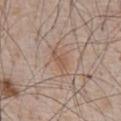Impression: Imaged during a routine full-body skin examination; the lesion was not biopsied and no histopathology is available. Image and clinical context: A male subject aged 63–67. Automated image analysis of the tile measured a footprint of about 4 mm² and a shape-asymmetry score of about 0.5 (0 = symmetric). It also reported a border-irregularity index near 5.5/10, a within-lesion color-variation index near 1.5/10, and radial color variation of about 0.5. It also reported an automated nevus-likeness rating near 15 out of 100 and a detector confidence of about 100 out of 100 that the crop contains a lesion. Longest diameter approximately 3.5 mm. Captured under white-light illumination. The lesion is located on the chest. A 15 mm crop from a total-body photograph taken for skin-cancer surveillance.